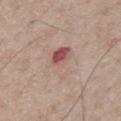{
  "biopsy_status": "not biopsied; imaged during a skin examination",
  "image": {
    "source": "total-body photography crop",
    "field_of_view_mm": 15
  },
  "lesion_size": {
    "long_diameter_mm_approx": 4.5
  },
  "site": "chest",
  "patient": {
    "sex": "male",
    "age_approx": 75
  },
  "automated_metrics": {
    "vs_skin_contrast_norm": 5.5
  },
  "lighting": "white-light"
}